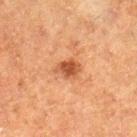Q: Is there a histopathology result?
A: total-body-photography surveillance lesion; no biopsy
Q: Patient demographics?
A: female, aged 48–52
Q: Where on the body is the lesion?
A: the right lower leg
Q: What kind of image is this?
A: 15 mm crop, total-body photography
Q: What lighting was used for the tile?
A: cross-polarized illumination
Q: What did automated image analysis measure?
A: a classifier nevus-likeness of about 90/100 and a detector confidence of about 100 out of 100 that the crop contains a lesion